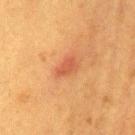A female subject, about 55 years old.
The lesion is on the mid back.
The lesion's longest dimension is about 2.5 mm.
The tile uses cross-polarized illumination.
A 15 mm crop from a total-body photograph taken for skin-cancer surveillance.